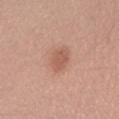Cropped from a whole-body photographic skin survey; the tile spans about 15 mm. A female patient, aged 33 to 37. The total-body-photography lesion software estimated a lesion-to-skin contrast of about 6 (normalized; higher = more distinct). This is a white-light tile. Located on the right forearm.